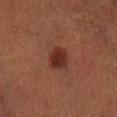Captured during whole-body skin photography for melanoma surveillance; the lesion was not biopsied. The patient is a male in their 50s. Automated image analysis of the tile measured a border-irregularity rating of about 2/10 and a within-lesion color-variation index near 2.5/10. The analysis additionally found a classifier nevus-likeness of about 100/100 and lesion-presence confidence of about 100/100. This is a cross-polarized tile. A 15 mm close-up tile from a total-body photography series done for melanoma screening. The lesion's longest dimension is about 3 mm. From the right lower leg.